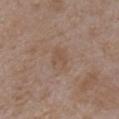No biopsy was performed on this lesion — it was imaged during a full skin examination and was not determined to be concerning.
A 15 mm close-up tile from a total-body photography series done for melanoma screening.
Captured under white-light illumination.
A male patient, about 75 years old.
On the arm.